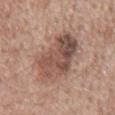Assessment: Part of a total-body skin-imaging series; this lesion was reviewed on a skin check and was not flagged for biopsy. Context: A 15 mm crop from a total-body photograph taken for skin-cancer surveillance. Automated image analysis of the tile measured a lesion color around L≈50 a*≈19 b*≈25 in CIELAB, a lesion–skin lightness drop of about 12, and a normalized border contrast of about 8.5. It also reported a classifier nevus-likeness of about 35/100 and a detector confidence of about 100 out of 100 that the crop contains a lesion. Measured at roughly 8 mm in maximum diameter. Imaged with white-light lighting. Located on the back. The patient is a male roughly 60 years of age.